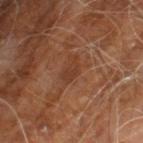patient:
  sex: male
  age_approx: 60
lesion_size:
  long_diameter_mm_approx: 3.0
image:
  source: total-body photography crop
  field_of_view_mm: 15
site: right leg